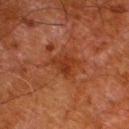Case summary:
- notes — total-body-photography surveillance lesion; no biopsy
- lesion size — ≈3 mm
- image-analysis metrics — a nevus-likeness score of about 0/100 and lesion-presence confidence of about 100/100
- lighting — cross-polarized illumination
- body site — the left lower leg
- image source — ~15 mm crop, total-body skin-cancer survey
- patient — male, roughly 80 years of age